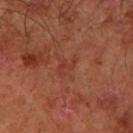Impression: No biopsy was performed on this lesion — it was imaged during a full skin examination and was not determined to be concerning. Background: A close-up tile cropped from a whole-body skin photograph, about 15 mm across. On the arm. A male patient in their 60s.